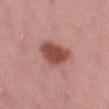The lesion was tiled from a total-body skin photograph and was not biopsied. About 5 mm across. The subject is a female aged 43–47. This is a white-light tile. Cropped from a total-body skin-imaging series; the visible field is about 15 mm. From the left thigh. Automated image analysis of the tile measured a classifier nevus-likeness of about 95/100 and a detector confidence of about 100 out of 100 that the crop contains a lesion.